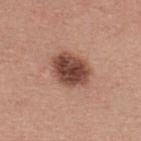{
  "biopsy_status": "not biopsied; imaged during a skin examination",
  "lighting": "white-light",
  "site": "upper back",
  "patient": {
    "sex": "male",
    "age_approx": 40
  },
  "image": {
    "source": "total-body photography crop",
    "field_of_view_mm": 15
  }
}